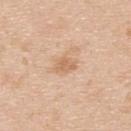This lesion was catalogued during total-body skin photography and was not selected for biopsy. The lesion is on the back. Approximately 2.5 mm at its widest. A 15 mm close-up tile from a total-body photography series done for melanoma screening. A male subject roughly 35 years of age.